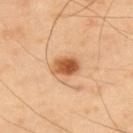The lesion was photographed on a routine skin check and not biopsied; there is no pathology result. The tile uses cross-polarized illumination. A male subject, aged 38–42. Located on the upper back. Cropped from a whole-body photographic skin survey; the tile spans about 15 mm.